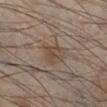Impression:
Imaged during a routine full-body skin examination; the lesion was not biopsied and no histopathology is available.
Background:
The lesion is located on the leg. A male subject in their mid-40s. A region of skin cropped from a whole-body photographic capture, roughly 15 mm wide. Measured at roughly 2.5 mm in maximum diameter.